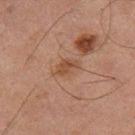Imaged during a routine full-body skin examination; the lesion was not biopsied and no histopathology is available. A 15 mm close-up extracted from a 3D total-body photography capture. This is a cross-polarized tile. A male subject aged approximately 65. From the right thigh.